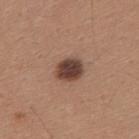The lesion was tiled from a total-body skin photograph and was not biopsied.
Cropped from a total-body skin-imaging series; the visible field is about 15 mm.
A male subject approximately 30 years of age.
Located on the upper back.
The lesion's longest dimension is about 3.5 mm.
The lesion-visualizer software estimated an area of roughly 7.5 mm² and two-axis asymmetry of about 0.15. The analysis additionally found a border-irregularity index near 1/10, internal color variation of about 4.5 on a 0–10 scale, and peripheral color asymmetry of about 1.5. It also reported a nevus-likeness score of about 75/100.
The tile uses white-light illumination.